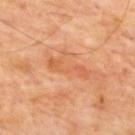Q: Is there a histopathology result?
A: imaged on a skin check; not biopsied
Q: Lesion size?
A: ~5 mm (longest diameter)
Q: Patient demographics?
A: male, aged 63–67
Q: What did automated image analysis measure?
A: a lesion color around L≈58 a*≈27 b*≈39 in CIELAB, roughly 7 lightness units darker than nearby skin, and a normalized lesion–skin contrast near 5.5; a border-irregularity rating of about 9/10, a within-lesion color-variation index near 3.5/10, and radial color variation of about 1; a nevus-likeness score of about 0/100 and lesion-presence confidence of about 100/100
Q: How was the tile lit?
A: cross-polarized illumination
Q: Lesion location?
A: the upper back
Q: What kind of image is this?
A: 15 mm crop, total-body photography